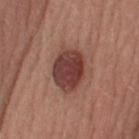{"lighting": "white-light", "image": {"source": "total-body photography crop", "field_of_view_mm": 15}, "automated_metrics": {"nevus_likeness_0_100": 95}, "site": "head or neck", "patient": {"sex": "male", "age_approx": 25}}